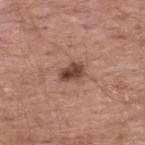Clinical impression:
The lesion was photographed on a routine skin check and not biopsied; there is no pathology result.
Context:
An algorithmic analysis of the crop reported a footprint of about 5 mm², an eccentricity of roughly 0.75, and a symmetry-axis asymmetry near 0.25. The software also gave a color-variation rating of about 4/10 and peripheral color asymmetry of about 1.5. The analysis additionally found a nevus-likeness score of about 80/100 and a lesion-detection confidence of about 100/100. The patient is a male in their mid-50s. This is a white-light tile. This image is a 15 mm lesion crop taken from a total-body photograph. From the upper back. Approximately 3 mm at its widest.